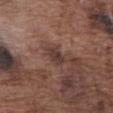| feature | finding |
|---|---|
| follow-up | no biopsy performed (imaged during a skin exam) |
| illumination | white-light illumination |
| image source | ~15 mm tile from a whole-body skin photo |
| site | the abdomen |
| lesion diameter | ≈2.5 mm |
| subject | male, about 75 years old |